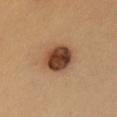Imaged during a routine full-body skin examination; the lesion was not biopsied and no histopathology is available. A female patient in their mid- to late 30s. A region of skin cropped from a whole-body photographic capture, roughly 15 mm wide. About 4 mm across. The lesion is located on the chest.